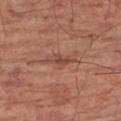{
  "biopsy_status": "not biopsied; imaged during a skin examination",
  "image": {
    "source": "total-body photography crop",
    "field_of_view_mm": 15
  },
  "site": "left upper arm",
  "patient": {
    "sex": "male",
    "age_approx": 65
  }
}